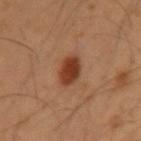Acquisition and patient details: Approximately 3 mm at its widest. A region of skin cropped from a whole-body photographic capture, roughly 15 mm wide. Captured under cross-polarized illumination. Located on the left upper arm. A male subject, aged approximately 55.